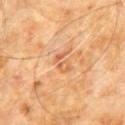• workup: no biopsy performed (imaged during a skin exam)
• subject: male, aged around 60
• anatomic site: the front of the torso
• image: ~15 mm crop, total-body skin-cancer survey